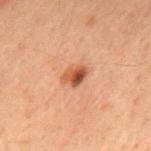follow-up=no biopsy performed (imaged during a skin exam)
size=about 3 mm
body site=the upper back
image source=~15 mm tile from a whole-body skin photo
patient=male, about 60 years old